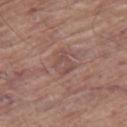The lesion was tiled from a total-body skin photograph and was not biopsied.
Longest diameter approximately 3 mm.
The lesion is on the right thigh.
A region of skin cropped from a whole-body photographic capture, roughly 15 mm wide.
The lesion-visualizer software estimated an average lesion color of about L≈49 a*≈20 b*≈22 (CIELAB), a lesion–skin lightness drop of about 6, and a normalized border contrast of about 4.5. The software also gave a border-irregularity index near 3.5/10, a within-lesion color-variation index near 3/10, and radial color variation of about 1.
A male subject, in their mid- to late 60s.
The tile uses white-light illumination.